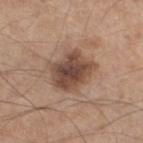  biopsy_status: not biopsied; imaged during a skin examination
  patient:
    sex: male
    age_approx: 55
  image:
    source: total-body photography crop
    field_of_view_mm: 15
  lighting: white-light
  site: leg